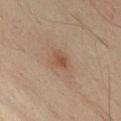The lesion was tiled from a total-body skin photograph and was not biopsied. Cropped from a total-body skin-imaging series; the visible field is about 15 mm. Imaged with cross-polarized lighting. A male patient, approximately 50 years of age. Longest diameter approximately 2.5 mm. From the chest.